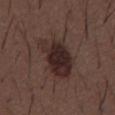No biopsy was performed on this lesion — it was imaged during a full skin examination and was not determined to be concerning.
Automated image analysis of the tile measured a border-irregularity index near 3.5/10 and peripheral color asymmetry of about 1.5. The software also gave a nevus-likeness score of about 75/100 and a lesion-detection confidence of about 100/100.
A lesion tile, about 15 mm wide, cut from a 3D total-body photograph.
The patient is a male in their 50s.
Captured under white-light illumination.
On the back.